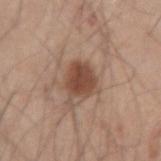• biopsy status · no biopsy performed (imaged during a skin exam)
• size · ≈4 mm
• body site · the arm
• image · ~15 mm crop, total-body skin-cancer survey
• subject · male, aged 43 to 47